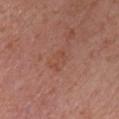follow-up — catalogued during a skin exam; not biopsied
lesion size — about 2.5 mm
anatomic site — the chest
subject — male, aged approximately 75
imaging modality — ~15 mm crop, total-body skin-cancer survey